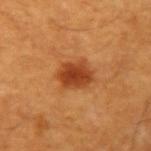An algorithmic analysis of the crop reported a lesion area of about 10 mm², an eccentricity of roughly 0.65, and a shape-asymmetry score of about 0.2 (0 = symmetric). The software also gave a mean CIELAB color near L≈41 a*≈27 b*≈38, a lesion–skin lightness drop of about 12, and a normalized border contrast of about 9. It also reported border irregularity of about 2.5 on a 0–10 scale, a color-variation rating of about 3.5/10, and radial color variation of about 1. The software also gave a nevus-likeness score of about 100/100 and a lesion-detection confidence of about 100/100.
A 15 mm close-up extracted from a 3D total-body photography capture.
The recorded lesion diameter is about 4 mm.
The lesion is on the left upper arm.
A male subject about 60 years old.